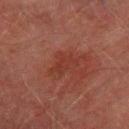Recorded during total-body skin imaging; not selected for excision or biopsy. The lesion is on the right thigh. Captured under cross-polarized illumination. The subject is a male about 75 years old. This image is a 15 mm lesion crop taken from a total-body photograph. Approximately 3 mm at its widest. Automated image analysis of the tile measured an average lesion color of about L≈30 a*≈24 b*≈24 (CIELAB) and a normalized lesion–skin contrast near 5. The analysis additionally found a border-irregularity index near 5.5/10, a within-lesion color-variation index near 1.5/10, and radial color variation of about 0.5.